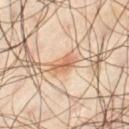Q: Was this lesion biopsied?
A: imaged on a skin check; not biopsied
Q: Where on the body is the lesion?
A: the leg
Q: What lighting was used for the tile?
A: cross-polarized illumination
Q: Patient demographics?
A: male, aged 38–42
Q: What is the lesion's diameter?
A: about 2.5 mm
Q: Automated lesion metrics?
A: a lesion area of about 3.5 mm², an eccentricity of roughly 0.8, and a symmetry-axis asymmetry near 0.4; a border-irregularity index near 4/10, a color-variation rating of about 2.5/10, and peripheral color asymmetry of about 1; an automated nevus-likeness rating near 100 out of 100 and a detector confidence of about 100 out of 100 that the crop contains a lesion
Q: What is the imaging modality?
A: ~15 mm crop, total-body skin-cancer survey